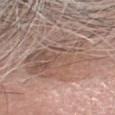imaging modality: 15 mm crop, total-body photography
site: the head or neck
patient: male, approximately 75 years of age
automated metrics: an area of roughly 36 mm² and an outline eccentricity of about 0.75 (0 = round, 1 = elongated); a lesion–skin lightness drop of about 8 and a normalized lesion–skin contrast near 5.5; lesion-presence confidence of about 80/100